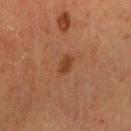Impression:
Captured during whole-body skin photography for melanoma surveillance; the lesion was not biopsied.
Clinical summary:
The lesion is on the right upper arm. A female patient roughly 60 years of age. The tile uses cross-polarized illumination. Automated tile analysis of the lesion measured a footprint of about 3.5 mm², a shape eccentricity near 0.8, and a shape-asymmetry score of about 0.15 (0 = symmetric). This image is a 15 mm lesion crop taken from a total-body photograph. Measured at roughly 2.5 mm in maximum diameter.